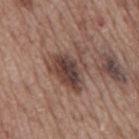A male subject aged 68–72. This image is a 15 mm lesion crop taken from a total-body photograph. The tile uses white-light illumination. The lesion is located on the mid back. The recorded lesion diameter is about 5.5 mm.